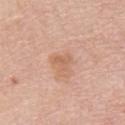A region of skin cropped from a whole-body photographic capture, roughly 15 mm wide. About 2.5 mm across. Automated image analysis of the tile measured roughly 7 lightness units darker than nearby skin and a normalized lesion–skin contrast near 5.5. The software also gave an automated nevus-likeness rating near 0 out of 100 and a detector confidence of about 100 out of 100 that the crop contains a lesion. Located on the back. A female patient, about 70 years old.